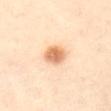notes = total-body-photography surveillance lesion; no biopsy
automated lesion analysis = a mean CIELAB color near L≈73 a*≈24 b*≈40, roughly 15 lightness units darker than nearby skin, and a normalized lesion–skin contrast near 8.5
subject = female, aged approximately 40
site = the abdomen
image = 15 mm crop, total-body photography
tile lighting = cross-polarized
lesion size = ≈3 mm